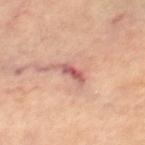{"biopsy_status": "not biopsied; imaged during a skin examination", "lesion_size": {"long_diameter_mm_approx": 3.5}, "patient": {"sex": "female", "age_approx": 65}, "image": {"source": "total-body photography crop", "field_of_view_mm": 15}, "site": "right lower leg"}